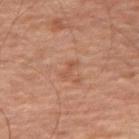Impression:
The lesion was tiled from a total-body skin photograph and was not biopsied.
Clinical summary:
The patient is a male in their 70s. The lesion's longest dimension is about 2.5 mm. Imaged with cross-polarized lighting. A 15 mm close-up extracted from a 3D total-body photography capture. From the right thigh. The total-body-photography lesion software estimated a mean CIELAB color near L≈39 a*≈18 b*≈25 and a lesion–skin lightness drop of about 5. And it measured a classifier nevus-likeness of about 0/100 and a lesion-detection confidence of about 100/100.